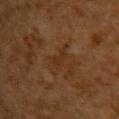The lesion was tiled from a total-body skin photograph and was not biopsied. Longest diameter approximately 2.5 mm. This image is a 15 mm lesion crop taken from a total-body photograph. On the upper back. A male subject approximately 60 years of age. The tile uses cross-polarized illumination. The lesion-visualizer software estimated a shape eccentricity near 0.85 and two-axis asymmetry of about 0.4.